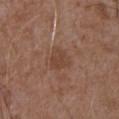{
  "biopsy_status": "not biopsied; imaged during a skin examination",
  "image": {
    "source": "total-body photography crop",
    "field_of_view_mm": 15
  },
  "patient": {
    "sex": "male",
    "age_approx": 75
  },
  "site": "abdomen"
}